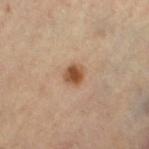  biopsy_status: not biopsied; imaged during a skin examination
  patient:
    sex: female
    age_approx: 65
  lesion_size:
    long_diameter_mm_approx: 2.5
  lighting: cross-polarized
  image:
    source: total-body photography crop
    field_of_view_mm: 15
  site: left thigh